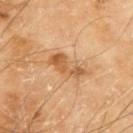No biopsy was performed on this lesion — it was imaged during a full skin examination and was not determined to be concerning. An algorithmic analysis of the crop reported a mean CIELAB color near L≈59 a*≈23 b*≈41, about 11 CIELAB-L* units darker than the surrounding skin, and a normalized lesion–skin contrast near 7.5. The software also gave a classifier nevus-likeness of about 0/100. This is a cross-polarized tile. Cropped from a whole-body photographic skin survey; the tile spans about 15 mm. The lesion is on the left upper arm. The patient is a male in their mid-60s.